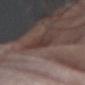Part of a total-body skin-imaging series; this lesion was reviewed on a skin check and was not flagged for biopsy.
Captured under white-light illumination.
A male patient, about 75 years old.
Longest diameter approximately 4 mm.
The lesion-visualizer software estimated an average lesion color of about L≈35 a*≈15 b*≈18 (CIELAB), roughly 7 lightness units darker than nearby skin, and a normalized border contrast of about 6.5. The software also gave a nevus-likeness score of about 0/100 and a detector confidence of about 95 out of 100 that the crop contains a lesion.
From the left forearm.
This image is a 15 mm lesion crop taken from a total-body photograph.